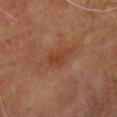acquisition = total-body-photography crop, ~15 mm field of view | lesion diameter = about 4 mm | lighting = cross-polarized illumination | anatomic site = the right lower leg | subject = male, roughly 70 years of age.